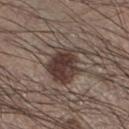Findings:
* notes · total-body-photography surveillance lesion; no biopsy
* automated metrics · a lesion area of about 14 mm², an outline eccentricity of about 0.65 (0 = round, 1 = elongated), and two-axis asymmetry of about 0.3; a lesion color around L≈37 a*≈14 b*≈19 in CIELAB, a lesion–skin lightness drop of about 12, and a normalized lesion–skin contrast near 10.5; a nevus-likeness score of about 90/100 and a lesion-detection confidence of about 100/100
* patient · male, aged approximately 35
* anatomic site · the left lower leg
* image · total-body-photography crop, ~15 mm field of view
* lesion size · about 5 mm
* illumination · white-light